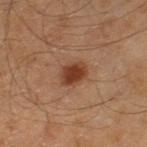{"lighting": "cross-polarized", "patient": {"sex": "male", "age_approx": 60}, "lesion_size": {"long_diameter_mm_approx": 3.0}, "image": {"source": "total-body photography crop", "field_of_view_mm": 15}, "site": "left thigh"}